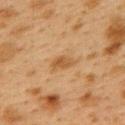| field | value |
|---|---|
| notes | catalogued during a skin exam; not biopsied |
| subject | female, aged approximately 40 |
| image source | ~15 mm tile from a whole-body skin photo |
| lesion diameter | ≈3 mm |
| lighting | cross-polarized illumination |
| anatomic site | the upper back |